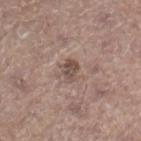body site: the right lower leg | subject: male, aged around 65 | diameter: ~2.5 mm (longest diameter) | imaging modality: total-body-photography crop, ~15 mm field of view | tile lighting: white-light.